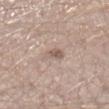Part of a total-body skin-imaging series; this lesion was reviewed on a skin check and was not flagged for biopsy.
Approximately 3 mm at its widest.
A 15 mm close-up extracted from a 3D total-body photography capture.
The lesion is located on the leg.
Captured under white-light illumination.
Automated tile analysis of the lesion measured a lesion area of about 4.5 mm², a shape eccentricity near 0.7, and two-axis asymmetry of about 0.25. The analysis additionally found a border-irregularity index near 3/10, a color-variation rating of about 3.5/10, and a peripheral color-asymmetry measure near 1.
A male subject approximately 30 years of age.